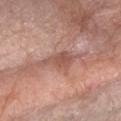{
  "biopsy_status": "not biopsied; imaged during a skin examination",
  "patient": {
    "sex": "female",
    "age_approx": 65
  },
  "lesion_size": {
    "long_diameter_mm_approx": 4.0
  },
  "image": {
    "source": "total-body photography crop",
    "field_of_view_mm": 15
  },
  "site": "right forearm"
}